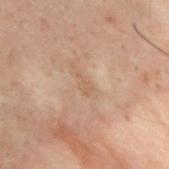Part of a total-body skin-imaging series; this lesion was reviewed on a skin check and was not flagged for biopsy. Located on the back. Captured under cross-polarized illumination. The lesion-visualizer software estimated an outline eccentricity of about 0.9 (0 = round, 1 = elongated) and two-axis asymmetry of about 0.35. It also reported an average lesion color of about L≈47 a*≈14 b*≈27 (CIELAB) and a lesion–skin lightness drop of about 5. It also reported a classifier nevus-likeness of about 0/100 and a detector confidence of about 100 out of 100 that the crop contains a lesion. A region of skin cropped from a whole-body photographic capture, roughly 15 mm wide. A male subject roughly 30 years of age.